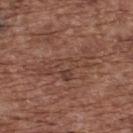patient:
  sex: male
  age_approx: 75
image:
  source: total-body photography crop
  field_of_view_mm: 15
site: upper back
lesion_size:
  long_diameter_mm_approx: 7.5
lighting: white-light
automated_metrics:
  area_mm2_approx: 13.0
  eccentricity: 0.95
  shape_asymmetry: 0.4
  lesion_detection_confidence_0_100: 60